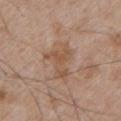• location: the chest
• subject: male, aged 63–67
• image: total-body-photography crop, ~15 mm field of view